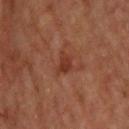Q: Is there a histopathology result?
A: catalogued during a skin exam; not biopsied
Q: How was the tile lit?
A: cross-polarized
Q: What kind of image is this?
A: ~15 mm tile from a whole-body skin photo
Q: What did automated image analysis measure?
A: a lesion area of about 4 mm², a shape eccentricity near 0.65, and a symmetry-axis asymmetry near 0.25; a nevus-likeness score of about 5/100 and a detector confidence of about 100 out of 100 that the crop contains a lesion
Q: Lesion size?
A: about 2.5 mm
Q: What is the anatomic site?
A: the upper back
Q: Who is the patient?
A: male, aged 68 to 72